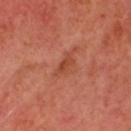The lesion was tiled from a total-body skin photograph and was not biopsied.
On the head or neck.
A male patient aged 58–62.
The tile uses cross-polarized illumination.
A region of skin cropped from a whole-body photographic capture, roughly 15 mm wide.
Automated image analysis of the tile measured a lesion area of about 3.5 mm², a shape eccentricity near 0.95, and two-axis asymmetry of about 0.35.